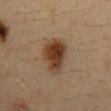Imaged during a routine full-body skin examination; the lesion was not biopsied and no histopathology is available.